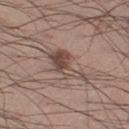Notes:
• workup: catalogued during a skin exam; not biopsied
• lighting: white-light illumination
• subject: male, roughly 55 years of age
• image source: ~15 mm crop, total-body skin-cancer survey
• lesion diameter: ≈5 mm
• location: the right thigh